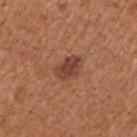Q: Was this lesion biopsied?
A: no biopsy performed (imaged during a skin exam)
Q: What kind of image is this?
A: total-body-photography crop, ~15 mm field of view
Q: Where on the body is the lesion?
A: the left upper arm
Q: Who is the patient?
A: female, aged 38–42